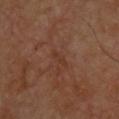| feature | finding |
|---|---|
| automated metrics | a footprint of about 2 mm², an eccentricity of roughly 0.95, and a symmetry-axis asymmetry near 0.25; roughly 5 lightness units darker than nearby skin and a lesion-to-skin contrast of about 5 (normalized; higher = more distinct); border irregularity of about 3.5 on a 0–10 scale, a within-lesion color-variation index near 0/10, and a peripheral color-asymmetry measure near 0; a nevus-likeness score of about 0/100 and lesion-presence confidence of about 95/100 |
| image | ~15 mm crop, total-body skin-cancer survey |
| lesion size | ≈2.5 mm |
| lighting | cross-polarized illumination |
| subject | male, in their 60s |
| location | the back |